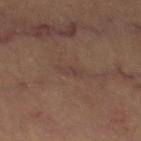workup — total-body-photography surveillance lesion; no biopsy | diameter — ≈3 mm | patient — female, in their mid- to late 60s | automated lesion analysis — a lesion area of about 2.5 mm²; a lesion–skin lightness drop of about 4 and a lesion-to-skin contrast of about 4.5 (normalized; higher = more distinct); a border-irregularity rating of about 5.5/10, internal color variation of about 0 on a 0–10 scale, and peripheral color asymmetry of about 0; a classifier nevus-likeness of about 0/100 | illumination — cross-polarized illumination | body site — the left thigh | imaging modality — ~15 mm tile from a whole-body skin photo.